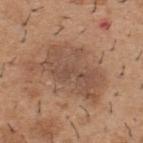Assessment:
The lesion was photographed on a routine skin check and not biopsied; there is no pathology result.
Background:
A male subject, aged 38 to 42. The lesion is located on the back. Cropped from a total-body skin-imaging series; the visible field is about 15 mm. Captured under white-light illumination.